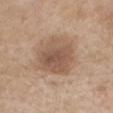The lesion was photographed on a routine skin check and not biopsied; there is no pathology result.
Cropped from a total-body skin-imaging series; the visible field is about 15 mm.
A female subject aged around 60.
On the mid back.